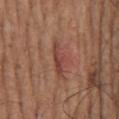biopsy status: catalogued during a skin exam; not biopsied
image: total-body-photography crop, ~15 mm field of view
lighting: white-light
body site: the mid back
patient: male, approximately 75 years of age
lesion diameter: ~4.5 mm (longest diameter)